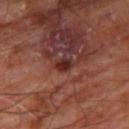biopsy status=imaged on a skin check; not biopsied
image source=total-body-photography crop, ~15 mm field of view
subject=aged approximately 65
body site=the leg